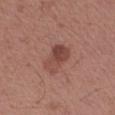Captured during whole-body skin photography for melanoma surveillance; the lesion was not biopsied. Captured under white-light illumination. A male patient in their mid-40s. About 4.5 mm across. A 15 mm close-up extracted from a 3D total-body photography capture. On the left forearm.